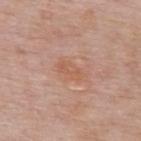The lesion is on the back. A lesion tile, about 15 mm wide, cut from a 3D total-body photograph. A female subject, aged 48–52.